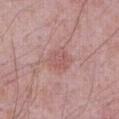follow-up: no biopsy performed (imaged during a skin exam); location: the abdomen; subject: male, aged around 70; image: ~15 mm crop, total-body skin-cancer survey.